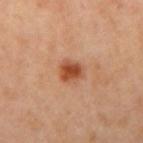This lesion was catalogued during total-body skin photography and was not selected for biopsy.
A roughly 15 mm field-of-view crop from a total-body skin photograph.
This is a cross-polarized tile.
The lesion is located on the right upper arm.
The lesion-visualizer software estimated a lesion area of about 5.5 mm² and a symmetry-axis asymmetry near 0.25. It also reported a lesion–skin lightness drop of about 12. It also reported a border-irregularity index near 2/10, internal color variation of about 3.5 on a 0–10 scale, and radial color variation of about 1. And it measured a nevus-likeness score of about 100/100 and a detector confidence of about 100 out of 100 that the crop contains a lesion.
The lesion's longest dimension is about 3 mm.
A male subject, aged 43–47.